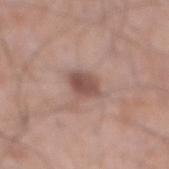Impression:
This lesion was catalogued during total-body skin photography and was not selected for biopsy.
Acquisition and patient details:
The tile uses white-light illumination. On the abdomen. A male subject aged approximately 70. A lesion tile, about 15 mm wide, cut from a 3D total-body photograph.